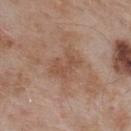Case summary:
– biopsy status · catalogued during a skin exam; not biopsied
– tile lighting · white-light
– image source · total-body-photography crop, ~15 mm field of view
– size · ≈4 mm
– TBP lesion metrics · a lesion color around L≈51 a*≈19 b*≈29 in CIELAB, roughly 7 lightness units darker than nearby skin, and a lesion-to-skin contrast of about 5 (normalized; higher = more distinct); a border-irregularity index near 6.5/10, a color-variation rating of about 1/10, and peripheral color asymmetry of about 0.5; a nevus-likeness score of about 0/100 and a lesion-detection confidence of about 100/100
– anatomic site · the back
– patient · male, in their mid- to late 50s